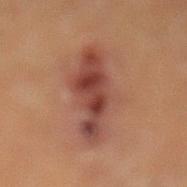Q: Illumination type?
A: cross-polarized illumination
Q: How was this image acquired?
A: ~15 mm tile from a whole-body skin photo
Q: Where on the body is the lesion?
A: the leg
Q: Patient demographics?
A: male, about 60 years old
Q: Lesion size?
A: about 9.5 mm
Q: Automated lesion metrics?
A: a detector confidence of about 95 out of 100 that the crop contains a lesion